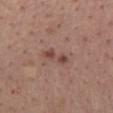Notes:
* workup · total-body-photography surveillance lesion; no biopsy
* location · the mid back
* patient · female, in their 50s
* image source · 15 mm crop, total-body photography
* size · ≈3.5 mm
* illumination · white-light illumination